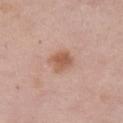biopsy status: no biopsy performed (imaged during a skin exam); site: the chest; illumination: white-light; acquisition: ~15 mm crop, total-body skin-cancer survey; subject: female, about 65 years old.